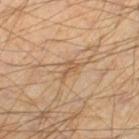Q: Was a biopsy performed?
A: catalogued during a skin exam; not biopsied
Q: Who is the patient?
A: male, aged around 45
Q: How was the tile lit?
A: cross-polarized illumination
Q: How was this image acquired?
A: ~15 mm tile from a whole-body skin photo
Q: Lesion location?
A: the right lower leg